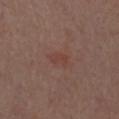| feature | finding |
|---|---|
| biopsy status | total-body-photography surveillance lesion; no biopsy |
| tile lighting | white-light illumination |
| patient | male, approximately 70 years of age |
| location | the chest |
| image source | ~15 mm crop, total-body skin-cancer survey |
| diameter | ≈2.5 mm |